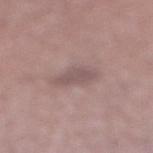Context: A 15 mm crop from a total-body photograph taken for skin-cancer surveillance. The lesion is located on the right lower leg. Captured under white-light illumination. A male patient roughly 65 years of age. Automated image analysis of the tile measured a lesion area of about 5 mm² and a shape-asymmetry score of about 0.3 (0 = symmetric). The software also gave an average lesion color of about L≈52 a*≈17 b*≈18 (CIELAB), about 8 CIELAB-L* units darker than the surrounding skin, and a normalized lesion–skin contrast near 6. And it measured a border-irregularity index near 3/10 and internal color variation of about 1 on a 0–10 scale. The software also gave an automated nevus-likeness rating near 0 out of 100 and lesion-presence confidence of about 95/100.